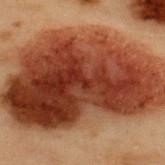automated_metrics:
  cielab_L: 32
  cielab_a: 24
  cielab_b: 28
  vs_skin_darker_L: 17.0
  vs_skin_contrast_norm: 14.0
  nevus_likeness_0_100: 100
  lesion_detection_confidence_0_100: 100
patient:
  sex: male
  age_approx: 55
site: upper back
lesion_size:
  long_diameter_mm_approx: 16.0
image:
  source: total-body photography crop
  field_of_view_mm: 15